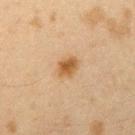Clinical impression: Part of a total-body skin-imaging series; this lesion was reviewed on a skin check and was not flagged for biopsy. Context: Located on the arm. Cropped from a total-body skin-imaging series; the visible field is about 15 mm. The recorded lesion diameter is about 3 mm. The tile uses cross-polarized illumination. The subject is a female roughly 40 years of age.